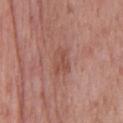Clinical impression:
The lesion was tiled from a total-body skin photograph and was not biopsied.
Background:
Imaged with white-light lighting. On the chest. This image is a 15 mm lesion crop taken from a total-body photograph. The lesion's longest dimension is about 3 mm. The subject is a male in their mid- to late 50s. The lesion-visualizer software estimated a lesion area of about 5.5 mm², an outline eccentricity of about 0.75 (0 = round, 1 = elongated), and a shape-asymmetry score of about 0.3 (0 = symmetric). And it measured a lesion color around L≈50 a*≈24 b*≈27 in CIELAB and a normalized lesion–skin contrast near 5.5. The analysis additionally found an automated nevus-likeness rating near 0 out of 100 and lesion-presence confidence of about 100/100.